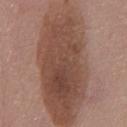Q: What is the anatomic site?
A: the front of the torso
Q: What are the patient's age and sex?
A: male, in their mid-50s
Q: What is the imaging modality?
A: total-body-photography crop, ~15 mm field of view